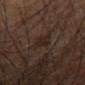Clinical impression: No biopsy was performed on this lesion — it was imaged during a full skin examination and was not determined to be concerning. Background: Automated image analysis of the tile measured an average lesion color of about L≈22 a*≈13 b*≈20 (CIELAB), a lesion–skin lightness drop of about 5, and a normalized lesion–skin contrast near 6.5. The software also gave a border-irregularity index near 3/10, a color-variation rating of about 1/10, and radial color variation of about 0. The software also gave an automated nevus-likeness rating near 5 out of 100 and lesion-presence confidence of about 85/100. Captured under cross-polarized illumination. A close-up tile cropped from a whole-body skin photograph, about 15 mm across. The lesion is on the arm. The patient is a male in their mid- to late 60s.